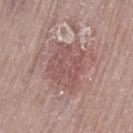Located on the left thigh. Cropped from a total-body skin-imaging series; the visible field is about 15 mm. The patient is a female in their mid-60s.